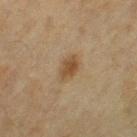Recorded during total-body skin imaging; not selected for excision or biopsy.
The total-body-photography lesion software estimated a footprint of about 5.5 mm², a shape eccentricity near 0.8, and a symmetry-axis asymmetry near 0.25. The software also gave a normalized lesion–skin contrast near 7.5. And it measured a classifier nevus-likeness of about 90/100 and lesion-presence confidence of about 100/100.
About 3.5 mm across.
A lesion tile, about 15 mm wide, cut from a 3D total-body photograph.
The subject is a female roughly 55 years of age.
Imaged with cross-polarized lighting.
The lesion is on the leg.